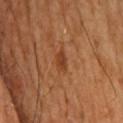Imaged during a routine full-body skin examination; the lesion was not biopsied and no histopathology is available. Imaged with cross-polarized lighting. A lesion tile, about 15 mm wide, cut from a 3D total-body photograph. The subject is a male aged 68 to 72. On the head or neck.